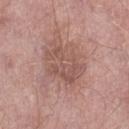Case summary:
– notes — total-body-photography surveillance lesion; no biopsy
– imaging modality — ~15 mm tile from a whole-body skin photo
– automated lesion analysis — a border-irregularity rating of about 5/10 and a peripheral color-asymmetry measure near 1
– diameter — ≈5.5 mm
– subject — male, in their mid-50s
– anatomic site — the right lower leg